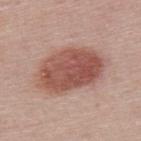This lesion was catalogued during total-body skin photography and was not selected for biopsy. The tile uses white-light illumination. From the upper back. Measured at roughly 8 mm in maximum diameter. A female subject, in their 50s. A region of skin cropped from a whole-body photographic capture, roughly 15 mm wide.